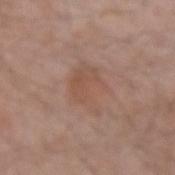biopsy_status: not biopsied; imaged during a skin examination
image:
  source: total-body photography crop
  field_of_view_mm: 15
patient:
  sex: male
  age_approx: 55
site: left forearm
lesion_size:
  long_diameter_mm_approx: 5.0